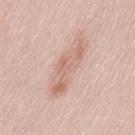The lesion is located on the back.
An algorithmic analysis of the crop reported an average lesion color of about L≈66 a*≈19 b*≈27 (CIELAB) and about 9 CIELAB-L* units darker than the surrounding skin. It also reported a nevus-likeness score of about 0/100 and a lesion-detection confidence of about 90/100.
The patient is a female about 65 years old.
Imaged with white-light lighting.
A 15 mm crop from a total-body photograph taken for skin-cancer surveillance.